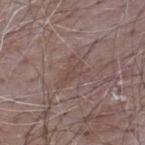Q: Lesion size?
A: about 3 mm
Q: Who is the patient?
A: male, aged 63–67
Q: How was this image acquired?
A: ~15 mm tile from a whole-body skin photo
Q: Where on the body is the lesion?
A: the upper back